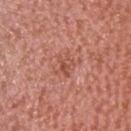The lesion was photographed on a routine skin check and not biopsied; there is no pathology result.
The subject is a female in their 50s.
A roughly 15 mm field-of-view crop from a total-body skin photograph.
This is a white-light tile.
About 2.5 mm across.
From the head or neck.